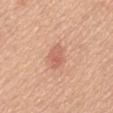biopsy status = catalogued during a skin exam; not biopsied | anatomic site = the back | subject = female, aged around 55 | lesion diameter = ≈3 mm | TBP lesion metrics = a classifier nevus-likeness of about 65/100 and lesion-presence confidence of about 100/100 | imaging modality = ~15 mm tile from a whole-body skin photo.